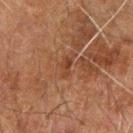Imaged during a routine full-body skin examination; the lesion was not biopsied and no histopathology is available. Captured under cross-polarized illumination. A lesion tile, about 15 mm wide, cut from a 3D total-body photograph. The lesion is located on the left arm. A male patient, aged approximately 60. Longest diameter approximately 2.5 mm.